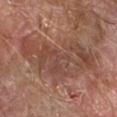Clinical impression:
This lesion was catalogued during total-body skin photography and was not selected for biopsy.
Background:
The subject is a male about 70 years old. On the right forearm. A lesion tile, about 15 mm wide, cut from a 3D total-body photograph. This is a cross-polarized tile. Longest diameter approximately 11 mm.